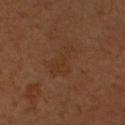notes = catalogued during a skin exam; not biopsied | illumination = cross-polarized | subject = male, aged approximately 50 | image source = ~15 mm tile from a whole-body skin photo | location = the head or neck | lesion diameter = ≈4 mm.